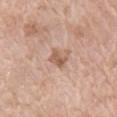<record>
  <biopsy_status>not biopsied; imaged during a skin examination</biopsy_status>
  <image>
    <source>total-body photography crop</source>
    <field_of_view_mm>15</field_of_view_mm>
  </image>
  <patient>
    <sex>female</sex>
    <age_approx>75</age_approx>
  </patient>
  <site>right upper arm</site>
</record>